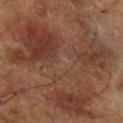No biopsy was performed on this lesion — it was imaged during a full skin examination and was not determined to be concerning.
Approximately 15 mm at its widest.
Imaged with cross-polarized lighting.
A roughly 15 mm field-of-view crop from a total-body skin photograph.
A male patient roughly 70 years of age.
Located on the right lower leg.